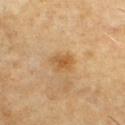workup: no biopsy performed (imaged during a skin exam); site: the chest; subject: male, in their 60s; imaging modality: ~15 mm crop, total-body skin-cancer survey; illumination: cross-polarized.